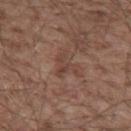Notes:
– notes: imaged on a skin check; not biopsied
– patient: male, approximately 55 years of age
– diameter: ~3.5 mm (longest diameter)
– site: the mid back
– image source: ~15 mm crop, total-body skin-cancer survey